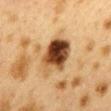<record>
  <biopsy_status>not biopsied; imaged during a skin examination</biopsy_status>
  <lesion_size>
    <long_diameter_mm_approx>5.5</long_diameter_mm_approx>
  </lesion_size>
  <image>
    <source>total-body photography crop</source>
    <field_of_view_mm>15</field_of_view_mm>
  </image>
  <site>mid back</site>
  <lighting>cross-polarized</lighting>
  <patient>
    <sex>female</sex>
    <age_approx>40</age_approx>
  </patient>
  <automated_metrics>
    <area_mm2_approx>15.0</area_mm2_approx>
    <eccentricity>0.7</eccentricity>
  </automated_metrics>
</record>